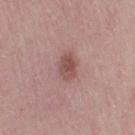Imaged during a routine full-body skin examination; the lesion was not biopsied and no histopathology is available. From the right thigh. Automated tile analysis of the lesion measured an area of roughly 5 mm², an eccentricity of roughly 0.7, and two-axis asymmetry of about 0.2. The software also gave a mean CIELAB color near L≈50 a*≈21 b*≈23 and a normalized lesion–skin contrast near 8. The analysis additionally found a nevus-likeness score of about 25/100 and a lesion-detection confidence of about 100/100. Captured under white-light illumination. A roughly 15 mm field-of-view crop from a total-body skin photograph. A female patient aged 48 to 52. Measured at roughly 3 mm in maximum diameter.